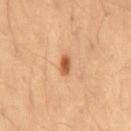{"lighting": "cross-polarized", "image": {"source": "total-body photography crop", "field_of_view_mm": 15}, "patient": {"sex": "female", "age_approx": 35}, "site": "left thigh", "automated_metrics": {"area_mm2_approx": 3.0, "eccentricity": 0.9, "shape_asymmetry": 0.3, "nevus_likeness_0_100": 100, "lesion_detection_confidence_0_100": 100}}